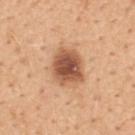| field | value |
|---|---|
| follow-up | no biopsy performed (imaged during a skin exam) |
| diameter | ~4 mm (longest diameter) |
| subject | male, aged approximately 50 |
| image source | ~15 mm tile from a whole-body skin photo |
| site | the mid back |